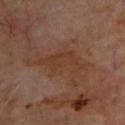{
  "biopsy_status": "not biopsied; imaged during a skin examination",
  "lesion_size": {
    "long_diameter_mm_approx": 6.5
  },
  "site": "upper back",
  "image": {
    "source": "total-body photography crop",
    "field_of_view_mm": 15
  },
  "patient": {
    "sex": "male",
    "age_approx": 70
  }
}